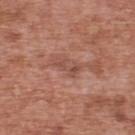This lesion was catalogued during total-body skin photography and was not selected for biopsy. Approximately 3.5 mm at its widest. Captured under white-light illumination. The lesion is on the upper back. A 15 mm close-up tile from a total-body photography series done for melanoma screening. The subject is a male aged approximately 70.